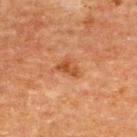size: about 3 mm
imaging modality: ~15 mm crop, total-body skin-cancer survey
subject: male, approximately 65 years of age
site: the upper back